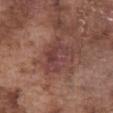Assessment: No biopsy was performed on this lesion — it was imaged during a full skin examination and was not determined to be concerning. Clinical summary: Captured under white-light illumination. Approximately 4.5 mm at its widest. The lesion is located on the abdomen. Cropped from a whole-body photographic skin survey; the tile spans about 15 mm. A male patient approximately 75 years of age.